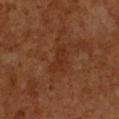| feature | finding |
|---|---|
| workup | imaged on a skin check; not biopsied |
| diameter | about 4 mm |
| lighting | cross-polarized illumination |
| patient | female, aged approximately 55 |
| location | the chest |
| imaging modality | 15 mm crop, total-body photography |